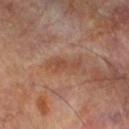The lesion was photographed on a routine skin check and not biopsied; there is no pathology result. A 15 mm close-up extracted from a 3D total-body photography capture. The total-body-photography lesion software estimated a lesion color around L≈47 a*≈21 b*≈30 in CIELAB and roughly 6 lightness units darker than nearby skin. A male patient aged 68 to 72. Longest diameter approximately 4 mm. Located on the left lower leg.